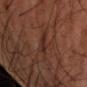Q: Is there a histopathology result?
A: no biopsy performed (imaged during a skin exam)
Q: How was the tile lit?
A: cross-polarized
Q: How large is the lesion?
A: ≈3.5 mm
Q: What is the anatomic site?
A: the left forearm
Q: What are the patient's age and sex?
A: male, roughly 55 years of age
Q: What did automated image analysis measure?
A: an average lesion color of about L≈26 a*≈18 b*≈23 (CIELAB), a lesion–skin lightness drop of about 6, and a normalized border contrast of about 6; peripheral color asymmetry of about 0.5
Q: What is the imaging modality?
A: 15 mm crop, total-body photography